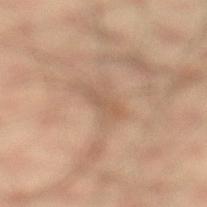Captured during whole-body skin photography for melanoma surveillance; the lesion was not biopsied. A male patient in their mid-30s. A roughly 15 mm field-of-view crop from a total-body skin photograph. The lesion is located on the left lower leg. Captured under cross-polarized illumination. Measured at roughly 3 mm in maximum diameter. An algorithmic analysis of the crop reported an area of roughly 2.5 mm², an outline eccentricity of about 0.95 (0 = round, 1 = elongated), and two-axis asymmetry of about 0.35. The software also gave an automated nevus-likeness rating near 0 out of 100 and lesion-presence confidence of about 80/100.